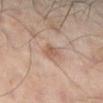follow-up = imaged on a skin check; not biopsied.